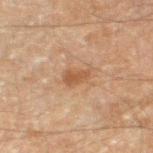Case summary:
– follow-up — total-body-photography surveillance lesion; no biopsy
– patient — male, aged around 60
– lesion size — ≈2.5 mm
– lighting — cross-polarized
– image — 15 mm crop, total-body photography
– body site — the left thigh